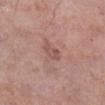notes — imaged on a skin check; not biopsied | imaging modality — total-body-photography crop, ~15 mm field of view | patient — male, about 70 years old | anatomic site — the right lower leg | lesion size — about 2.5 mm | image-analysis metrics — a nevus-likeness score of about 0/100 and a lesion-detection confidence of about 100/100.